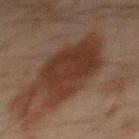– notes · imaged on a skin check; not biopsied
– anatomic site · the back
– imaging modality · ~15 mm tile from a whole-body skin photo
– subject · male, aged 43 to 47
– tile lighting · cross-polarized illumination
– diameter · ≈10 mm